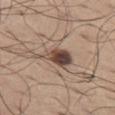{"biopsy_status": "not biopsied; imaged during a skin examination", "lesion_size": {"long_diameter_mm_approx": 4.5}, "image": {"source": "total-body photography crop", "field_of_view_mm": 15}, "automated_metrics": {"eccentricity": 0.8, "shape_asymmetry": 0.45, "cielab_L": 46, "cielab_a": 16, "cielab_b": 23, "vs_skin_darker_L": 16.0, "vs_skin_contrast_norm": 11.5, "border_irregularity_0_10": 4.5, "color_variation_0_10": 9.5, "peripheral_color_asymmetry": 3.0, "nevus_likeness_0_100": 95, "lesion_detection_confidence_0_100": 100}, "patient": {"sex": "male", "age_approx": 65}, "lighting": "white-light", "site": "left thigh"}